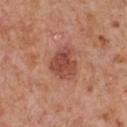follow-up: total-body-photography surveillance lesion; no biopsy
size: ≈4 mm
TBP lesion metrics: a lesion–skin lightness drop of about 11 and a normalized border contrast of about 8
image: total-body-photography crop, ~15 mm field of view
subject: male, in their mid- to late 60s
site: the chest